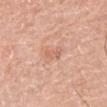Impression:
Recorded during total-body skin imaging; not selected for excision or biopsy.
Background:
The lesion-visualizer software estimated a lesion color around L≈64 a*≈24 b*≈31 in CIELAB and roughly 7 lightness units darker than nearby skin. And it measured a border-irregularity index near 4/10, internal color variation of about 0.5 on a 0–10 scale, and a peripheral color-asymmetry measure near 0. On the right upper arm. A male subject, aged approximately 50. Cropped from a total-body skin-imaging series; the visible field is about 15 mm. Measured at roughly 3 mm in maximum diameter.